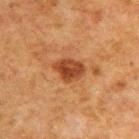The lesion was photographed on a routine skin check and not biopsied; there is no pathology result. Imaged with cross-polarized lighting. The lesion's longest dimension is about 3.5 mm. The patient is a male in their 50s. Automated tile analysis of the lesion measured a lesion area of about 7 mm², a shape eccentricity near 0.65, and two-axis asymmetry of about 0.2. And it measured an average lesion color of about L≈36 a*≈24 b*≈33 (CIELAB), a lesion–skin lightness drop of about 11, and a normalized border contrast of about 9.5. It also reported a border-irregularity rating of about 2/10, a color-variation rating of about 3.5/10, and a peripheral color-asymmetry measure near 1.5. It also reported a classifier nevus-likeness of about 70/100 and a lesion-detection confidence of about 100/100. A roughly 15 mm field-of-view crop from a total-body skin photograph. From the upper back.